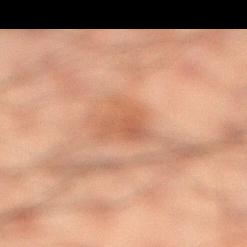Q: Was a biopsy performed?
A: total-body-photography surveillance lesion; no biopsy
Q: What is the anatomic site?
A: the right lower leg
Q: What kind of image is this?
A: 15 mm crop, total-body photography
Q: How large is the lesion?
A: ≈3.5 mm
Q: Automated lesion metrics?
A: border irregularity of about 4 on a 0–10 scale and radial color variation of about 1
Q: How was the tile lit?
A: cross-polarized illumination
Q: Who is the patient?
A: male, aged 48 to 52